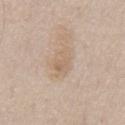No biopsy was performed on this lesion — it was imaged during a full skin examination and was not determined to be concerning. A male subject aged 63–67. Cropped from a whole-body photographic skin survey; the tile spans about 15 mm. On the chest. Captured under white-light illumination. An algorithmic analysis of the crop reported a footprint of about 7.5 mm², an eccentricity of roughly 0.75, and a shape-asymmetry score of about 0.25 (0 = symmetric). And it measured a mean CIELAB color near L≈64 a*≈14 b*≈29 and a normalized border contrast of about 5. And it measured a nevus-likeness score of about 0/100 and lesion-presence confidence of about 100/100.